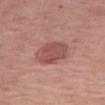biopsy_status: not biopsied; imaged during a skin examination
lesion_size:
  long_diameter_mm_approx: 4.0
lighting: white-light
site: right thigh
image:
  source: total-body photography crop
  field_of_view_mm: 15
automated_metrics:
  border_irregularity_0_10: 1.5
  color_variation_0_10: 3.0
  peripheral_color_asymmetry: 1.0
  nevus_likeness_0_100: 85
  lesion_detection_confidence_0_100: 100
patient:
  sex: female
  age_approx: 65